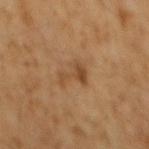follow-up: no biopsy performed (imaged during a skin exam)
subject: male, approximately 60 years of age
lighting: cross-polarized illumination
automated lesion analysis: a lesion area of about 5 mm², a shape eccentricity near 0.8, and two-axis asymmetry of about 0.65; a lesion color around L≈42 a*≈18 b*≈33 in CIELAB, roughly 7 lightness units darker than nearby skin, and a normalized lesion–skin contrast near 6.5; border irregularity of about 7.5 on a 0–10 scale and a within-lesion color-variation index near 1.5/10; a nevus-likeness score of about 0/100
anatomic site: the mid back
acquisition: ~15 mm tile from a whole-body skin photo
diameter: ≈3.5 mm